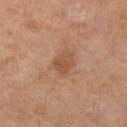Q: Is there a histopathology result?
A: total-body-photography surveillance lesion; no biopsy
Q: Lesion size?
A: ≈3 mm
Q: Where on the body is the lesion?
A: the left lower leg
Q: What kind of image is this?
A: 15 mm crop, total-body photography
Q: Patient demographics?
A: female, in their mid- to late 60s
Q: Automated lesion metrics?
A: an area of roughly 5 mm², a shape eccentricity near 0.7, and a shape-asymmetry score of about 0.2 (0 = symmetric); an average lesion color of about L≈48 a*≈21 b*≈31 (CIELAB), about 8 CIELAB-L* units darker than the surrounding skin, and a normalized border contrast of about 6
Q: What lighting was used for the tile?
A: cross-polarized illumination